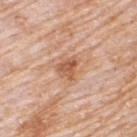Assessment:
Part of a total-body skin-imaging series; this lesion was reviewed on a skin check and was not flagged for biopsy.
Context:
The total-body-photography lesion software estimated a lesion area of about 5 mm². The analysis additionally found radial color variation of about 1. And it measured lesion-presence confidence of about 100/100. A 15 mm close-up extracted from a 3D total-body photography capture. Imaged with white-light lighting. A male patient, aged 78 to 82. The lesion is located on the upper back.